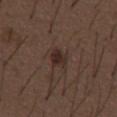Q: Is there a histopathology result?
A: total-body-photography surveillance lesion; no biopsy
Q: Lesion size?
A: ≈2.5 mm
Q: Where on the body is the lesion?
A: the abdomen
Q: Patient demographics?
A: male, approximately 50 years of age
Q: What lighting was used for the tile?
A: white-light
Q: What is the imaging modality?
A: total-body-photography crop, ~15 mm field of view